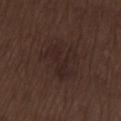Longest diameter approximately 5 mm.
A male subject in their 70s.
The total-body-photography lesion software estimated an area of roughly 11 mm² and a shape eccentricity near 0.75.
A close-up tile cropped from a whole-body skin photograph, about 15 mm across.
The lesion is located on the left thigh.